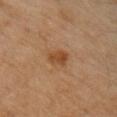Background: A male patient, approximately 60 years of age. Cropped from a total-body skin-imaging series; the visible field is about 15 mm. Located on the right upper arm. Captured under cross-polarized illumination.